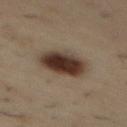Clinical impression:
The lesion was photographed on a routine skin check and not biopsied; there is no pathology result.
Image and clinical context:
The patient is a male in their mid- to late 50s. A roughly 15 mm field-of-view crop from a total-body skin photograph. Automated tile analysis of the lesion measured a mean CIELAB color near L≈34 a*≈14 b*≈22, a lesion–skin lightness drop of about 16, and a normalized border contrast of about 13.5. And it measured a border-irregularity index near 1.5/10, a within-lesion color-variation index near 7.5/10, and peripheral color asymmetry of about 2. On the back. Measured at roughly 5.5 mm in maximum diameter. Captured under cross-polarized illumination.